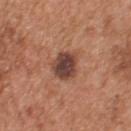Captured during whole-body skin photography for melanoma surveillance; the lesion was not biopsied. This is a white-light tile. Cropped from a whole-body photographic skin survey; the tile spans about 15 mm. A male subject, in their mid-50s. The recorded lesion diameter is about 4 mm. Automated tile analysis of the lesion measured a footprint of about 9 mm², a shape eccentricity near 0.55, and two-axis asymmetry of about 0.2. And it measured a lesion color around L≈43 a*≈21 b*≈26 in CIELAB, roughly 14 lightness units darker than nearby skin, and a lesion-to-skin contrast of about 11 (normalized; higher = more distinct). And it measured a within-lesion color-variation index near 5/10 and radial color variation of about 1.5. And it measured a lesion-detection confidence of about 100/100. Located on the back.